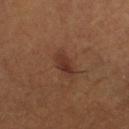Context:
Captured under cross-polarized illumination. Cropped from a whole-body photographic skin survey; the tile spans about 15 mm. A female subject about 80 years old. From the right lower leg.